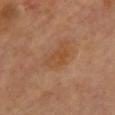Captured during whole-body skin photography for melanoma surveillance; the lesion was not biopsied.
A female subject, aged 38 to 42.
The tile uses cross-polarized illumination.
The lesion is located on the chest.
A roughly 15 mm field-of-view crop from a total-body skin photograph.